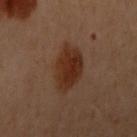| key | value |
|---|---|
| follow-up | imaged on a skin check; not biopsied |
| lesion diameter | ≈5 mm |
| tile lighting | cross-polarized |
| subject | female, aged approximately 60 |
| anatomic site | the left arm |
| image source | ~15 mm crop, total-body skin-cancer survey |
| image-analysis metrics | a footprint of about 13 mm²; a border-irregularity index near 2/10 and a within-lesion color-variation index near 3/10; an automated nevus-likeness rating near 95 out of 100 and lesion-presence confidence of about 100/100 |